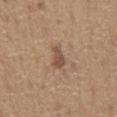Part of a total-body skin-imaging series; this lesion was reviewed on a skin check and was not flagged for biopsy.
On the abdomen.
A male subject aged 63–67.
A 15 mm crop from a total-body photograph taken for skin-cancer surveillance.